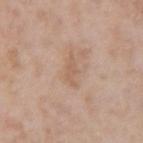The total-body-photography lesion software estimated an area of roughly 4 mm², a shape eccentricity near 0.9, and a symmetry-axis asymmetry near 0.45. It also reported a within-lesion color-variation index near 1/10 and a peripheral color-asymmetry measure near 0.5. A roughly 15 mm field-of-view crop from a total-body skin photograph. A male patient, roughly 60 years of age. About 3.5 mm across. Imaged with white-light lighting. Located on the right upper arm.